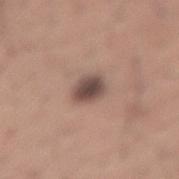Q: Was a biopsy performed?
A: imaged on a skin check; not biopsied
Q: Illumination type?
A: white-light illumination
Q: Lesion size?
A: ~3 mm (longest diameter)
Q: Where on the body is the lesion?
A: the lower back
Q: Patient demographics?
A: male, aged 43 to 47
Q: How was this image acquired?
A: ~15 mm crop, total-body skin-cancer survey